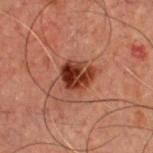Captured during whole-body skin photography for melanoma surveillance; the lesion was not biopsied. The tile uses cross-polarized illumination. The patient is a male aged approximately 60. From the chest. Longest diameter approximately 4 mm. A close-up tile cropped from a whole-body skin photograph, about 15 mm across.